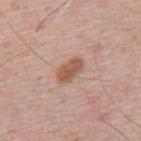The lesion was tiled from a total-body skin photograph and was not biopsied.